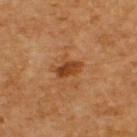follow-up: catalogued during a skin exam; not biopsied
lighting: cross-polarized illumination
patient: male, aged around 60
lesion size: ≈3.5 mm
imaging modality: total-body-photography crop, ~15 mm field of view
body site: the upper back